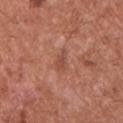| feature | finding |
|---|---|
| biopsy status | total-body-photography surveillance lesion; no biopsy |
| acquisition | ~15 mm crop, total-body skin-cancer survey |
| subject | male, roughly 75 years of age |
| anatomic site | the chest |
| lighting | white-light illumination |